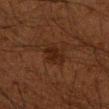Case summary:
* biopsy status: imaged on a skin check; not biopsied
* lesion diameter: about 2.5 mm
* acquisition: total-body-photography crop, ~15 mm field of view
* automated lesion analysis: a mean CIELAB color near L≈18 a*≈17 b*≈22, roughly 6 lightness units darker than nearby skin, and a normalized lesion–skin contrast near 8; a border-irregularity rating of about 3/10, internal color variation of about 1.5 on a 0–10 scale, and a peripheral color-asymmetry measure near 0.5
* lighting: cross-polarized illumination
* patient: male, aged 63 to 67
* anatomic site: the right upper arm